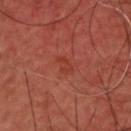Impression:
Captured during whole-body skin photography for melanoma surveillance; the lesion was not biopsied.
Acquisition and patient details:
On the back. A male patient, about 45 years old. Automated tile analysis of the lesion measured about 5 CIELAB-L* units darker than the surrounding skin. And it measured internal color variation of about 1 on a 0–10 scale and radial color variation of about 0.5. The software also gave a classifier nevus-likeness of about 0/100. Longest diameter approximately 2.5 mm. A region of skin cropped from a whole-body photographic capture, roughly 15 mm wide.